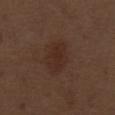Q: Was a biopsy performed?
A: total-body-photography surveillance lesion; no biopsy